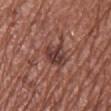Assessment: No biopsy was performed on this lesion — it was imaged during a full skin examination and was not determined to be concerning. Background: The tile uses white-light illumination. A female subject, in their mid- to late 60s. The lesion-visualizer software estimated a footprint of about 7 mm² and an eccentricity of roughly 0.45. And it measured a within-lesion color-variation index near 5/10 and a peripheral color-asymmetry measure near 2. The software also gave an automated nevus-likeness rating near 0 out of 100 and a lesion-detection confidence of about 100/100. From the chest. A lesion tile, about 15 mm wide, cut from a 3D total-body photograph.